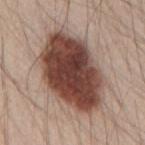Part of a total-body skin-imaging series; this lesion was reviewed on a skin check and was not flagged for biopsy. A male patient, in their mid-60s. This is a white-light tile. Cropped from a total-body skin-imaging series; the visible field is about 15 mm. Automated tile analysis of the lesion measured an average lesion color of about L≈44 a*≈20 b*≈24 (CIELAB), roughly 20 lightness units darker than nearby skin, and a lesion-to-skin contrast of about 14.5 (normalized; higher = more distinct). The software also gave a border-irregularity rating of about 2/10, internal color variation of about 7 on a 0–10 scale, and a peripheral color-asymmetry measure near 2. The software also gave a nevus-likeness score of about 100/100 and lesion-presence confidence of about 100/100. The recorded lesion diameter is about 10 mm. The lesion is located on the upper back.